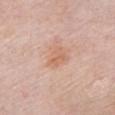Cropped from a whole-body photographic skin survey; the tile spans about 15 mm. Automated image analysis of the tile measured a footprint of about 5.5 mm². The software also gave a lesion color around L≈64 a*≈22 b*≈32 in CIELAB, about 7 CIELAB-L* units darker than the surrounding skin, and a normalized lesion–skin contrast near 5.5. The analysis additionally found a border-irregularity index near 2.5/10, internal color variation of about 3 on a 0–10 scale, and peripheral color asymmetry of about 1. About 3 mm across. The tile uses white-light illumination. The lesion is located on the chest. A female patient about 70 years old.